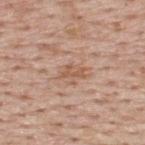Image and clinical context: From the upper back. This image is a 15 mm lesion crop taken from a total-body photograph. A male patient aged around 75.